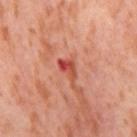The lesion was photographed on a routine skin check and not biopsied; there is no pathology result. Captured under cross-polarized illumination. A female subject aged approximately 55. A region of skin cropped from a whole-body photographic capture, roughly 15 mm wide. Measured at roughly 3 mm in maximum diameter. On the right thigh. Automated tile analysis of the lesion measured a mean CIELAB color near L≈52 a*≈36 b*≈34 and a lesion–skin lightness drop of about 12. And it measured a border-irregularity index near 4/10, internal color variation of about 7 on a 0–10 scale, and radial color variation of about 2. The software also gave a classifier nevus-likeness of about 0/100 and a detector confidence of about 100 out of 100 that the crop contains a lesion.